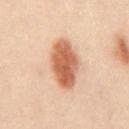biopsy status — total-body-photography surveillance lesion; no biopsy
image source — ~15 mm crop, total-body skin-cancer survey
automated lesion analysis — roughly 14 lightness units darker than nearby skin and a lesion-to-skin contrast of about 10.5 (normalized; higher = more distinct); a border-irregularity index near 1.5/10 and peripheral color asymmetry of about 1.5
lighting — cross-polarized
patient — male, approximately 50 years of age
location — the abdomen